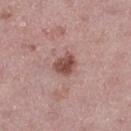  biopsy_status: not biopsied; imaged during a skin examination
  patient:
    sex: female
    age_approx: 45
  image:
    source: total-body photography crop
    field_of_view_mm: 15
  site: left thigh
  lighting: white-light